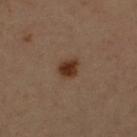Clinical summary:
Automated image analysis of the tile measured a footprint of about 5 mm². The software also gave a nevus-likeness score of about 100/100 and a lesion-detection confidence of about 100/100. A 15 mm close-up extracted from a 3D total-body photography capture. A male subject aged 63–67. On the right upper arm. Longest diameter approximately 2.5 mm.